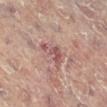| feature | finding |
|---|---|
| follow-up | no biopsy performed (imaged during a skin exam) |
| site | the left leg |
| tile lighting | cross-polarized |
| imaging modality | ~15 mm crop, total-body skin-cancer survey |
| patient | female, aged 78 to 82 |
| lesion diameter | ≈3.5 mm |